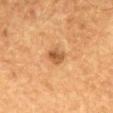  biopsy_status: not biopsied; imaged during a skin examination
  lesion_size:
    long_diameter_mm_approx: 2.5
  automated_metrics:
    color_variation_0_10: 3.0
    peripheral_color_asymmetry: 1.0
    nevus_likeness_0_100: 45
    lesion_detection_confidence_0_100: 100
  image:
    source: total-body photography crop
    field_of_view_mm: 15
  patient:
    sex: male
    age_approx: 65
  lighting: cross-polarized
  site: mid back